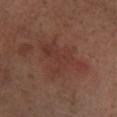Q: What lighting was used for the tile?
A: cross-polarized illumination
Q: What is the imaging modality?
A: total-body-photography crop, ~15 mm field of view
Q: What is the anatomic site?
A: the leg
Q: What are the patient's age and sex?
A: male, approximately 65 years of age